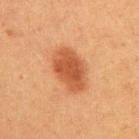Q: Was this lesion biopsied?
A: total-body-photography surveillance lesion; no biopsy
Q: Automated lesion metrics?
A: an outline eccentricity of about 0.8 (0 = round, 1 = elongated) and a shape-asymmetry score of about 0.15 (0 = symmetric); border irregularity of about 2 on a 0–10 scale
Q: What is the imaging modality?
A: total-body-photography crop, ~15 mm field of view
Q: Who is the patient?
A: male, aged approximately 40
Q: What lighting was used for the tile?
A: cross-polarized illumination
Q: Where on the body is the lesion?
A: the arm